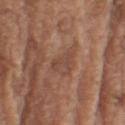Q: Was a biopsy performed?
A: imaged on a skin check; not biopsied
Q: Lesion size?
A: ~3 mm (longest diameter)
Q: Automated lesion metrics?
A: a lesion color around L≈45 a*≈19 b*≈28 in CIELAB and roughly 6 lightness units darker than nearby skin; a classifier nevus-likeness of about 0/100
Q: Patient demographics?
A: male, aged 73–77
Q: What lighting was used for the tile?
A: white-light
Q: What kind of image is this?
A: ~15 mm tile from a whole-body skin photo
Q: Lesion location?
A: the right upper arm